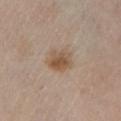Assessment:
Part of a total-body skin-imaging series; this lesion was reviewed on a skin check and was not flagged for biopsy.
Context:
Longest diameter approximately 3.5 mm. This is a cross-polarized tile. The patient is a female aged approximately 45. A 15 mm close-up tile from a total-body photography series done for melanoma screening. The lesion is on the leg.